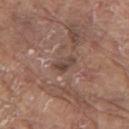Assessment:
The lesion was tiled from a total-body skin photograph and was not biopsied.
Acquisition and patient details:
On the right upper arm. The patient is a male about 80 years old. A 15 mm crop from a total-body photograph taken for skin-cancer surveillance. Longest diameter approximately 3 mm. The tile uses white-light illumination.